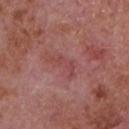Recorded during total-body skin imaging; not selected for excision or biopsy. The lesion is on the chest. The patient is a male in their mid- to late 60s. A lesion tile, about 15 mm wide, cut from a 3D total-body photograph. Automated image analysis of the tile measured roughly 6 lightness units darker than nearby skin and a normalized lesion–skin contrast near 5. The analysis additionally found a lesion-detection confidence of about 90/100. Captured under white-light illumination. Measured at roughly 3.5 mm in maximum diameter.